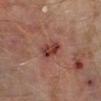follow-up: catalogued during a skin exam; not biopsied
subject: male, in their 60s
lesion size: about 3.5 mm
illumination: cross-polarized illumination
imaging modality: ~15 mm crop, total-body skin-cancer survey
TBP lesion metrics: a color-variation rating of about 5/10 and radial color variation of about 1.5
site: the right lower leg